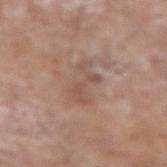{
  "biopsy_status": "not biopsied; imaged during a skin examination",
  "lighting": "white-light",
  "site": "left forearm",
  "patient": {
    "sex": "male",
    "age_approx": 80
  },
  "automated_metrics": {
    "border_irregularity_0_10": 6.5,
    "peripheral_color_asymmetry": 0.5
  },
  "image": {
    "source": "total-body photography crop",
    "field_of_view_mm": 15
  }
}